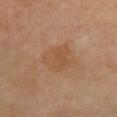• workup: no biopsy performed (imaged during a skin exam)
• lesion diameter: about 4 mm
• image source: ~15 mm crop, total-body skin-cancer survey
• subject: female, roughly 40 years of age
• location: the chest
• lighting: cross-polarized illumination